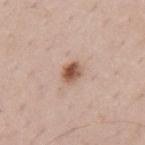| field | value |
|---|---|
| follow-up | no biopsy performed (imaged during a skin exam) |
| subject | male, aged 68–72 |
| lesion size | ≈2.5 mm |
| acquisition | ~15 mm tile from a whole-body skin photo |
| site | the mid back |
| tile lighting | white-light |